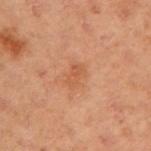Q: Was a biopsy performed?
A: imaged on a skin check; not biopsied
Q: What is the anatomic site?
A: the left upper arm
Q: What is the imaging modality?
A: ~15 mm crop, total-body skin-cancer survey
Q: Patient demographics?
A: female, in their mid- to late 50s
Q: Automated lesion metrics?
A: a border-irregularity rating of about 3/10 and a peripheral color-asymmetry measure near 0.5; a classifier nevus-likeness of about 5/100 and a lesion-detection confidence of about 100/100
Q: Lesion size?
A: ≈2.5 mm
Q: Illumination type?
A: cross-polarized illumination